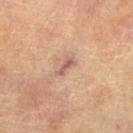Clinical impression:
This lesion was catalogued during total-body skin photography and was not selected for biopsy.
Background:
The tile uses cross-polarized illumination. Automated tile analysis of the lesion measured a lesion color around L≈49 a*≈19 b*≈23 in CIELAB, a lesion–skin lightness drop of about 9, and a normalized lesion–skin contrast near 7.5. And it measured a border-irregularity rating of about 3.5/10, a color-variation rating of about 0/10, and a peripheral color-asymmetry measure near 0. And it measured a detector confidence of about 90 out of 100 that the crop contains a lesion. The subject is a female aged approximately 80. The lesion's longest dimension is about 2.5 mm. The lesion is on the left lower leg. A region of skin cropped from a whole-body photographic capture, roughly 15 mm wide.